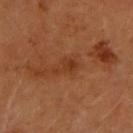workup: no biopsy performed (imaged during a skin exam)
lighting: cross-polarized illumination
location: the arm
automated metrics: an area of roughly 4.5 mm² and a shape eccentricity near 0.8; a mean CIELAB color near L≈39 a*≈26 b*≈36, a lesion–skin lightness drop of about 7, and a lesion-to-skin contrast of about 6 (normalized; higher = more distinct); a classifier nevus-likeness of about 0/100 and lesion-presence confidence of about 100/100
lesion size: about 3 mm
image source: 15 mm crop, total-body photography
subject: female, aged approximately 70